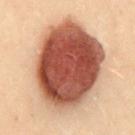Clinical impression:
Imaged during a routine full-body skin examination; the lesion was not biopsied and no histopathology is available.
Clinical summary:
The patient is a female aged 28 to 32. Approximately 11.5 mm at its widest. The lesion is located on the back. The total-body-photography lesion software estimated an area of roughly 70 mm² and two-axis asymmetry of about 0.1. And it measured a mean CIELAB color near L≈43 a*≈21 b*≈26 and a lesion–skin lightness drop of about 20. It also reported a classifier nevus-likeness of about 100/100 and lesion-presence confidence of about 100/100. Cropped from a whole-body photographic skin survey; the tile spans about 15 mm.